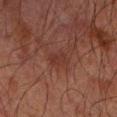Impression:
This lesion was catalogued during total-body skin photography and was not selected for biopsy.
Image and clinical context:
Imaged with cross-polarized lighting. Measured at roughly 3 mm in maximum diameter. A 15 mm crop from a total-body photograph taken for skin-cancer surveillance. A male patient aged approximately 65. An algorithmic analysis of the crop reported a lesion-detection confidence of about 100/100. The lesion is on the right forearm.